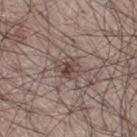Q: What lighting was used for the tile?
A: white-light illumination
Q: Automated lesion metrics?
A: a border-irregularity rating of about 3/10 and internal color variation of about 5 on a 0–10 scale; an automated nevus-likeness rating near 70 out of 100
Q: Patient demographics?
A: male, aged approximately 30
Q: What is the lesion's diameter?
A: ~3 mm (longest diameter)
Q: Lesion location?
A: the right thigh
Q: What kind of image is this?
A: total-body-photography crop, ~15 mm field of view